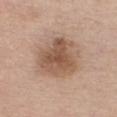workup — no biopsy performed (imaged during a skin exam)
size — ~5.5 mm (longest diameter)
automated metrics — about 11 CIELAB-L* units darker than the surrounding skin and a normalized lesion–skin contrast near 8
body site — the chest
subject — female, aged approximately 55
image source — ~15 mm tile from a whole-body skin photo